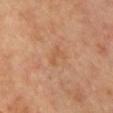Clinical impression: Imaged during a routine full-body skin examination; the lesion was not biopsied and no histopathology is available. Background: Located on the chest. The lesion-visualizer software estimated a lesion area of about 4 mm², a shape eccentricity near 0.7, and a shape-asymmetry score of about 0.45 (0 = symmetric). The software also gave a border-irregularity index near 4.5/10, a within-lesion color-variation index near 1/10, and radial color variation of about 0. A female patient, aged approximately 40. A roughly 15 mm field-of-view crop from a total-body skin photograph. Approximately 3 mm at its widest.